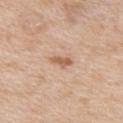biopsy_status: not biopsied; imaged during a skin examination
image:
  source: total-body photography crop
  field_of_view_mm: 15
patient:
  sex: male
  age_approx: 65
site: chest